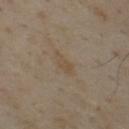No biopsy was performed on this lesion — it was imaged during a full skin examination and was not determined to be concerning.
A roughly 15 mm field-of-view crop from a total-body skin photograph.
A male patient, approximately 55 years of age.
Automated image analysis of the tile measured an outline eccentricity of about 0.9 (0 = round, 1 = elongated) and two-axis asymmetry of about 0.35. And it measured an average lesion color of about L≈47 a*≈12 b*≈28 (CIELAB), a lesion–skin lightness drop of about 5, and a normalized lesion–skin contrast near 5.5.
From the chest.
Captured under cross-polarized illumination.